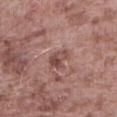notes: no biopsy performed (imaged during a skin exam)
acquisition: ~15 mm crop, total-body skin-cancer survey
anatomic site: the abdomen
TBP lesion metrics: a lesion color around L≈47 a*≈21 b*≈22 in CIELAB, roughly 10 lightness units darker than nearby skin, and a normalized lesion–skin contrast near 7.5; border irregularity of about 3.5 on a 0–10 scale, internal color variation of about 5.5 on a 0–10 scale, and radial color variation of about 2; a nevus-likeness score of about 0/100 and a detector confidence of about 100 out of 100 that the crop contains a lesion
lesion diameter: ≈2.5 mm
subject: male, aged 73 to 77